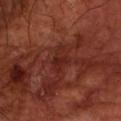biopsy_status: not biopsied; imaged during a skin examination
image:
  source: total-body photography crop
  field_of_view_mm: 15
site: left forearm
lighting: cross-polarized
patient:
  sex: male
  age_approx: 70